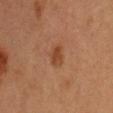Acquisition and patient details:
This is a cross-polarized tile. The patient is a male aged around 60. A roughly 15 mm field-of-view crop from a total-body skin photograph. The lesion is on the front of the torso.